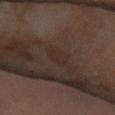Assessment:
This lesion was catalogued during total-body skin photography and was not selected for biopsy.
Image and clinical context:
The lesion is on the right forearm. The lesion's longest dimension is about 2.5 mm. This image is a 15 mm lesion crop taken from a total-body photograph. A male subject, aged 58 to 62. The total-body-photography lesion software estimated an eccentricity of roughly 0.85 and a shape-asymmetry score of about 0.25 (0 = symmetric). The software also gave border irregularity of about 2.5 on a 0–10 scale, internal color variation of about 1.5 on a 0–10 scale, and radial color variation of about 0.5. And it measured a detector confidence of about 100 out of 100 that the crop contains a lesion.